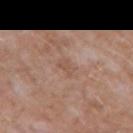| feature | finding |
|---|---|
| follow-up | catalogued during a skin exam; not biopsied |
| patient | male, roughly 60 years of age |
| lesion size | ~2.5 mm (longest diameter) |
| site | the chest |
| image source | ~15 mm tile from a whole-body skin photo |